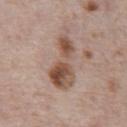Q: Was a biopsy performed?
A: total-body-photography surveillance lesion; no biopsy
Q: Where on the body is the lesion?
A: the abdomen
Q: What are the patient's age and sex?
A: male, approximately 70 years of age
Q: Automated lesion metrics?
A: a lesion area of about 17 mm² and a shape-asymmetry score of about 0.35 (0 = symmetric); a mean CIELAB color near L≈52 a*≈18 b*≈27, roughly 12 lightness units darker than nearby skin, and a lesion-to-skin contrast of about 8.5 (normalized; higher = more distinct); a nevus-likeness score of about 5/100 and lesion-presence confidence of about 100/100
Q: What is the imaging modality?
A: ~15 mm crop, total-body skin-cancer survey
Q: Lesion size?
A: ~8 mm (longest diameter)
Q: How was the tile lit?
A: white-light